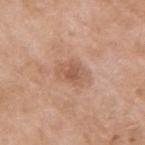biopsy status: imaged on a skin check; not biopsied
subject: female, roughly 75 years of age
location: the right upper arm
diameter: ~2.5 mm (longest diameter)
imaging modality: 15 mm crop, total-body photography